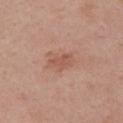Clinical impression: Imaged during a routine full-body skin examination; the lesion was not biopsied and no histopathology is available. Clinical summary: Approximately 2.5 mm at its widest. Captured under white-light illumination. A female patient aged approximately 55. From the arm. A 15 mm close-up extracted from a 3D total-body photography capture.